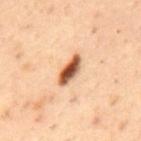This lesion was catalogued during total-body skin photography and was not selected for biopsy. Imaged with cross-polarized lighting. Cropped from a whole-body photographic skin survey; the tile spans about 15 mm. The subject is a male aged around 55. Approximately 4 mm at its widest. The lesion is located on the mid back.